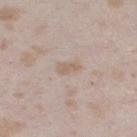Clinical impression: No biopsy was performed on this lesion — it was imaged during a full skin examination and was not determined to be concerning. Image and clinical context: The lesion is located on the right thigh. Approximately 3 mm at its widest. A female subject, aged around 25. A 15 mm close-up extracted from a 3D total-body photography capture.